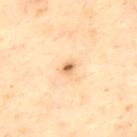No biopsy was performed on this lesion — it was imaged during a full skin examination and was not determined to be concerning. A 15 mm close-up tile from a total-body photography series done for melanoma screening. Approximately 1.5 mm at its widest. On the upper back. Imaged with cross-polarized lighting. The total-body-photography lesion software estimated a footprint of about 1.5 mm², an eccentricity of roughly 0.55, and a shape-asymmetry score of about 0.3 (0 = symmetric). The analysis additionally found a mean CIELAB color near L≈67 a*≈21 b*≈39, a lesion–skin lightness drop of about 15, and a normalized lesion–skin contrast near 8.5. The analysis additionally found a nevus-likeness score of about 85/100 and a lesion-detection confidence of about 100/100. A male subject in their mid- to late 40s.